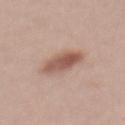Findings:
• biopsy status — catalogued during a skin exam; not biopsied
• lesion size — ≈5 mm
• anatomic site — the back
• TBP lesion metrics — a lesion area of about 9.5 mm², an outline eccentricity of about 0.9 (0 = round, 1 = elongated), and a symmetry-axis asymmetry near 0.1; a color-variation rating of about 4.5/10; a classifier nevus-likeness of about 95/100 and a lesion-detection confidence of about 100/100
• subject — female, roughly 40 years of age
• illumination — white-light
• image — ~15 mm crop, total-body skin-cancer survey